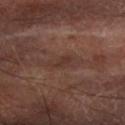Notes:
* subject: male, in their 70s
* body site: the arm
* image: total-body-photography crop, ~15 mm field of view
* illumination: cross-polarized illumination